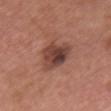{"biopsy_status": "not biopsied; imaged during a skin examination", "patient": {"sex": "female", "age_approx": 60}, "lesion_size": {"long_diameter_mm_approx": 4.5}, "site": "front of the torso", "image": {"source": "total-body photography crop", "field_of_view_mm": 15}, "lighting": "white-light"}